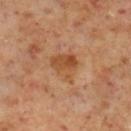<tbp_lesion>
<image>
  <source>total-body photography crop</source>
  <field_of_view_mm>15</field_of_view_mm>
</image>
<site>right lower leg</site>
<patient>
  <sex>male</sex>
  <age_approx>60</age_approx>
</patient>
<lesion_size>
  <long_diameter_mm_approx>3.5</long_diameter_mm_approx>
</lesion_size>
<lighting>cross-polarized</lighting>
<automated_metrics>
  <area_mm2_approx>9.0</area_mm2_approx>
  <shape_asymmetry>0.35</shape_asymmetry>
  <cielab_L>46</cielab_L>
  <cielab_a>22</cielab_a>
  <cielab_b>35</cielab_b>
  <vs_skin_darker_L>8.0</vs_skin_darker_L>
  <vs_skin_contrast_norm>7.0</vs_skin_contrast_norm>
  <border_irregularity_0_10>3.5</border_irregularity_0_10>
  <color_variation_0_10>5.5</color_variation_0_10>
  <peripheral_color_asymmetry>2.0</peripheral_color_asymmetry>
</automated_metrics>
</tbp_lesion>